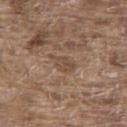workup = total-body-photography surveillance lesion; no biopsy | lesion diameter = about 3 mm | acquisition = 15 mm crop, total-body photography | TBP lesion metrics = an automated nevus-likeness rating near 0 out of 100 and a lesion-detection confidence of about 60/100 | illumination = white-light illumination | subject = male, aged 78 to 82 | body site = the upper back.